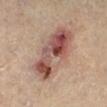Impression: Part of a total-body skin-imaging series; this lesion was reviewed on a skin check and was not flagged for biopsy. Acquisition and patient details: Imaged with cross-polarized lighting. A 15 mm crop from a total-body photograph taken for skin-cancer surveillance. Automated image analysis of the tile measured a mean CIELAB color near L≈52 a*≈22 b*≈25, about 14 CIELAB-L* units darker than the surrounding skin, and a lesion-to-skin contrast of about 9.5 (normalized; higher = more distinct). It also reported a border-irregularity rating of about 2.5/10 and a peripheral color-asymmetry measure near 4.5. The lesion is located on the right lower leg. A male subject in their mid- to late 60s.